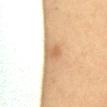Clinical impression:
Part of a total-body skin-imaging series; this lesion was reviewed on a skin check and was not flagged for biopsy.
Context:
Cropped from a total-body skin-imaging series; the visible field is about 15 mm. Measured at roughly 3 mm in maximum diameter. The patient is a female aged around 60. The lesion is located on the chest. Automated image analysis of the tile measured a lesion area of about 6.5 mm² and a shape eccentricity near 0.7. The software also gave a border-irregularity index near 2/10, a within-lesion color-variation index near 4/10, and radial color variation of about 1. The software also gave a nevus-likeness score of about 0/100. This is a cross-polarized tile.